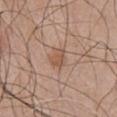Context: This image is a 15 mm lesion crop taken from a total-body photograph. A male patient, aged 48–52. The recorded lesion diameter is about 3 mm. From the chest. The tile uses white-light illumination.